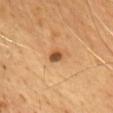Imaged during a routine full-body skin examination; the lesion was not biopsied and no histopathology is available.
A 15 mm crop from a total-body photograph taken for skin-cancer surveillance.
A male subject aged around 65.
The tile uses cross-polarized illumination.
Longest diameter approximately 2 mm.
From the chest.